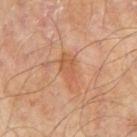Part of a total-body skin-imaging series; this lesion was reviewed on a skin check and was not flagged for biopsy. A 15 mm crop from a total-body photograph taken for skin-cancer surveillance. Located on the right upper arm. An algorithmic analysis of the crop reported about 7 CIELAB-L* units darker than the surrounding skin and a lesion-to-skin contrast of about 5.5 (normalized; higher = more distinct). The software also gave border irregularity of about 3.5 on a 0–10 scale, internal color variation of about 3.5 on a 0–10 scale, and peripheral color asymmetry of about 1. It also reported a nevus-likeness score of about 10/100 and a detector confidence of about 100 out of 100 that the crop contains a lesion. The subject is a male about 65 years old. The tile uses cross-polarized illumination. The lesion's longest dimension is about 4 mm.